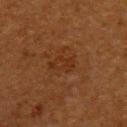  biopsy_status: not biopsied; imaged during a skin examination
  lighting: cross-polarized
  patient:
    sex: female
    age_approx: 50
  site: left upper arm
  image:
    source: total-body photography crop
    field_of_view_mm: 15